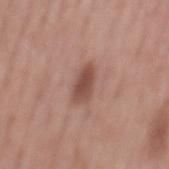Acquisition and patient details:
Located on the back. Longest diameter approximately 3.5 mm. Imaged with white-light lighting. A 15 mm close-up tile from a total-body photography series done for melanoma screening. Automated image analysis of the tile measured border irregularity of about 3 on a 0–10 scale, internal color variation of about 2.5 on a 0–10 scale, and radial color variation of about 1. It also reported a nevus-likeness score of about 95/100 and a lesion-detection confidence of about 100/100. A male subject, in their mid- to late 50s.